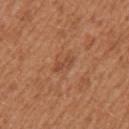The lesion is located on the left upper arm. Cropped from a total-body skin-imaging series; the visible field is about 15 mm. A female subject, aged around 40.